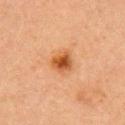Recorded during total-body skin imaging; not selected for excision or biopsy.
About 3 mm across.
The lesion is located on the right upper arm.
Automated tile analysis of the lesion measured a within-lesion color-variation index near 6/10 and radial color variation of about 1.5. The analysis additionally found an automated nevus-likeness rating near 95 out of 100 and lesion-presence confidence of about 100/100.
The tile uses cross-polarized illumination.
The subject is a female aged approximately 60.
Cropped from a whole-body photographic skin survey; the tile spans about 15 mm.